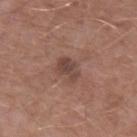{"biopsy_status": "not biopsied; imaged during a skin examination", "patient": {"sex": "male", "age_approx": 65}, "lighting": "white-light", "lesion_size": {"long_diameter_mm_approx": 3.0}, "site": "left upper arm", "image": {"source": "total-body photography crop", "field_of_view_mm": 15}}